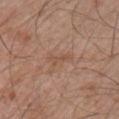notes — imaged on a skin check; not biopsied
anatomic site — the right upper arm
image source — 15 mm crop, total-body photography
subject — male, in their mid- to late 50s